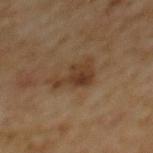The lesion was photographed on a routine skin check and not biopsied; there is no pathology result. Automated image analysis of the tile measured an average lesion color of about L≈33 a*≈16 b*≈28 (CIELAB), about 8 CIELAB-L* units darker than the surrounding skin, and a normalized lesion–skin contrast near 8. The analysis additionally found a border-irregularity index near 6.5/10, a within-lesion color-variation index near 4/10, and radial color variation of about 1.5. Captured under cross-polarized illumination. The patient is a male roughly 60 years of age. The lesion is located on the mid back. Cropped from a whole-body photographic skin survey; the tile spans about 15 mm. The recorded lesion diameter is about 4.5 mm.